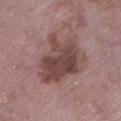Clinical impression:
The lesion was tiled from a total-body skin photograph and was not biopsied.
Background:
A female subject aged 68–72. Approximately 6.5 mm at its widest. A 15 mm close-up tile from a total-body photography series done for melanoma screening. Located on the left lower leg. The tile uses white-light illumination.